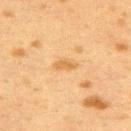biopsy_status: not biopsied; imaged during a skin examination
image:
  source: total-body photography crop
  field_of_view_mm: 15
patient:
  sex: female
  age_approx: 40
lighting: cross-polarized
site: upper back
automated_metrics:
  nevus_likeness_0_100: 5
  lesion_detection_confidence_0_100: 100
lesion_size:
  long_diameter_mm_approx: 2.5